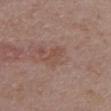No biopsy was performed on this lesion — it was imaged during a full skin examination and was not determined to be concerning. A close-up tile cropped from a whole-body skin photograph, about 15 mm across. The lesion-visualizer software estimated a lesion–skin lightness drop of about 6 and a lesion-to-skin contrast of about 5 (normalized; higher = more distinct). The software also gave a border-irregularity rating of about 3.5/10, a within-lesion color-variation index near 1.5/10, and radial color variation of about 0.5. The analysis additionally found a nevus-likeness score of about 0/100 and lesion-presence confidence of about 100/100. A female subject, approximately 65 years of age. Located on the upper back. This is a white-light tile.